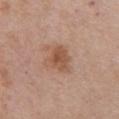Q: Was this lesion biopsied?
A: total-body-photography surveillance lesion; no biopsy